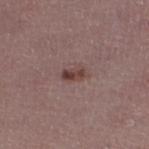workup: catalogued during a skin exam; not biopsied
patient: female, about 45 years old
illumination: white-light
lesion diameter: ~2.5 mm (longest diameter)
site: the left lower leg
acquisition: 15 mm crop, total-body photography